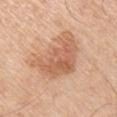Findings:
- follow-up — no biopsy performed (imaged during a skin exam)
- subject — male, roughly 55 years of age
- image source — total-body-photography crop, ~15 mm field of view
- lesion size — ≈6.5 mm
- site — the arm
- lighting — white-light illumination